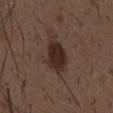Assessment: The lesion was tiled from a total-body skin photograph and was not biopsied. Context: A male patient, aged approximately 50. The tile uses white-light illumination. On the chest. A 15 mm close-up tile from a total-body photography series done for melanoma screening. The lesion-visualizer software estimated a lesion color around L≈27 a*≈15 b*≈20 in CIELAB and a normalized border contrast of about 11. And it measured a border-irregularity rating of about 2.5/10, a within-lesion color-variation index near 2.5/10, and peripheral color asymmetry of about 1.